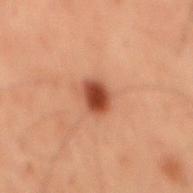Assessment:
Captured during whole-body skin photography for melanoma surveillance; the lesion was not biopsied.
Background:
The subject is a male aged 58 to 62. From the back. Measured at roughly 3 mm in maximum diameter. A 15 mm close-up tile from a total-body photography series done for melanoma screening. Imaged with cross-polarized lighting. Automated image analysis of the tile measured a lesion area of about 5.5 mm², a shape eccentricity near 0.6, and a symmetry-axis asymmetry near 0.15. The analysis additionally found a mean CIELAB color near L≈35 a*≈23 b*≈28, a lesion–skin lightness drop of about 13, and a lesion-to-skin contrast of about 11 (normalized; higher = more distinct). The analysis additionally found a within-lesion color-variation index near 2.5/10 and radial color variation of about 0.5.